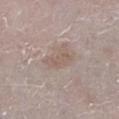This lesion was catalogued during total-body skin photography and was not selected for biopsy. The total-body-photography lesion software estimated an average lesion color of about L≈57 a*≈14 b*≈23 (CIELAB) and a lesion–skin lightness drop of about 7. And it measured a color-variation rating of about 1.5/10. The software also gave an automated nevus-likeness rating near 0 out of 100 and lesion-presence confidence of about 85/100. A male subject, aged 78–82. A 15 mm crop from a total-body photograph taken for skin-cancer surveillance. On the left lower leg.